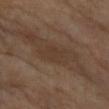Captured during whole-body skin photography for melanoma surveillance; the lesion was not biopsied.
This is a cross-polarized tile.
From the left forearm.
Approximately 4 mm at its widest.
A female patient, aged around 70.
A lesion tile, about 15 mm wide, cut from a 3D total-body photograph.